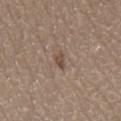biopsy status: no biopsy performed (imaged during a skin exam) | body site: the front of the torso | patient: male, in their 80s | size: about 2.5 mm | imaging modality: ~15 mm crop, total-body skin-cancer survey | lighting: white-light | TBP lesion metrics: a footprint of about 2.5 mm² and an outline eccentricity of about 0.9 (0 = round, 1 = elongated); a nevus-likeness score of about 0/100 and a detector confidence of about 100 out of 100 that the crop contains a lesion.